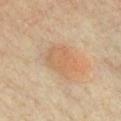Captured during whole-body skin photography for melanoma surveillance; the lesion was not biopsied. This is a cross-polarized tile. A 15 mm crop from a total-body photograph taken for skin-cancer surveillance. The subject is a male aged approximately 40. From the chest. The recorded lesion diameter is about 5.5 mm.